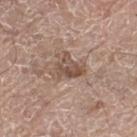Impression:
Part of a total-body skin-imaging series; this lesion was reviewed on a skin check and was not flagged for biopsy.
Background:
A male patient, in their mid- to late 70s. A region of skin cropped from a whole-body photographic capture, roughly 15 mm wide. From the left thigh. Approximately 4 mm at its widest. Automated image analysis of the tile measured a classifier nevus-likeness of about 0/100. Captured under white-light illumination.